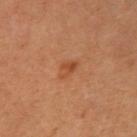The lesion was tiled from a total-body skin photograph and was not biopsied.
Automated tile analysis of the lesion measured a lesion area of about 3 mm², a shape eccentricity near 0.75, and two-axis asymmetry of about 0.4. The software also gave a border-irregularity index near 4/10 and peripheral color asymmetry of about 0.5. It also reported a lesion-detection confidence of about 100/100.
The patient is a female roughly 45 years of age.
A 15 mm close-up tile from a total-body photography series done for melanoma screening.
The lesion's longest dimension is about 2.5 mm.
Imaged with cross-polarized lighting.
The lesion is on the right upper arm.